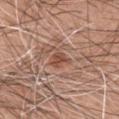| field | value |
|---|---|
| acquisition | ~15 mm tile from a whole-body skin photo |
| subject | male, about 60 years old |
| location | the left upper arm |
| lighting | white-light |
| size | ≈2.5 mm |